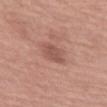Assessment:
This lesion was catalogued during total-body skin photography and was not selected for biopsy.
Acquisition and patient details:
The lesion is located on the right forearm. This is a white-light tile. Approximately 3 mm at its widest. Cropped from a total-body skin-imaging series; the visible field is about 15 mm. The subject is a female in their mid-60s.